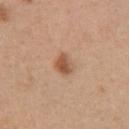biopsy_status: not biopsied; imaged during a skin examination
lesion_size:
  long_diameter_mm_approx: 2.5
automated_metrics:
  eccentricity: 0.55
  shape_asymmetry: 0.2
  border_irregularity_0_10: 1.5
  color_variation_0_10: 4.0
  peripheral_color_asymmetry: 1.0
image:
  source: total-body photography crop
  field_of_view_mm: 15
site: chest
patient:
  sex: female
  age_approx: 30